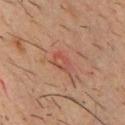Impression: The lesion was photographed on a routine skin check and not biopsied; there is no pathology result. Clinical summary: A 15 mm close-up tile from a total-body photography series done for melanoma screening. Measured at roughly 3 mm in maximum diameter. The patient is a male about 35 years old. The lesion-visualizer software estimated a lesion area of about 4.5 mm², an eccentricity of roughly 0.8, and a shape-asymmetry score of about 0.3 (0 = symmetric). It also reported a mean CIELAB color near L≈46 a*≈25 b*≈27, a lesion–skin lightness drop of about 6, and a normalized lesion–skin contrast near 4.5. The software also gave a border-irregularity rating of about 2.5/10, internal color variation of about 5 on a 0–10 scale, and peripheral color asymmetry of about 2. The software also gave a classifier nevus-likeness of about 0/100. The lesion is on the chest. Captured under cross-polarized illumination.